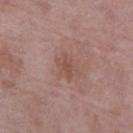The patient is a female aged 68 to 72. Automated tile analysis of the lesion measured a footprint of about 3.5 mm², an eccentricity of roughly 0.75, and a symmetry-axis asymmetry near 0.5. And it measured about 7 CIELAB-L* units darker than the surrounding skin and a normalized border contrast of about 5.5. The software also gave a border-irregularity rating of about 5/10 and a color-variation rating of about 1.5/10. The analysis additionally found a classifier nevus-likeness of about 0/100 and lesion-presence confidence of about 100/100. A roughly 15 mm field-of-view crop from a total-body skin photograph. Imaged with white-light lighting. From the leg.